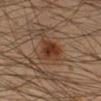notes=no biopsy performed (imaged during a skin exam) | subject=male, in their mid-40s | imaging modality=15 mm crop, total-body photography | location=the leg.